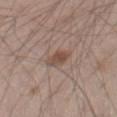Recorded during total-body skin imaging; not selected for excision or biopsy.